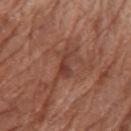Q: Is there a histopathology result?
A: imaged on a skin check; not biopsied
Q: Illumination type?
A: white-light illumination
Q: What are the patient's age and sex?
A: female, in their mid-70s
Q: What is the imaging modality?
A: 15 mm crop, total-body photography
Q: Lesion size?
A: ~3 mm (longest diameter)
Q: Automated lesion metrics?
A: a footprint of about 4 mm², a shape eccentricity near 0.9, and two-axis asymmetry of about 0.4; border irregularity of about 4.5 on a 0–10 scale
Q: What is the anatomic site?
A: the arm